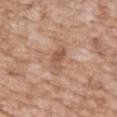Clinical impression: This lesion was catalogued during total-body skin photography and was not selected for biopsy. Acquisition and patient details: Located on the chest. A region of skin cropped from a whole-body photographic capture, roughly 15 mm wide. Automated image analysis of the tile measured a border-irregularity index near 3/10, a within-lesion color-variation index near 2.5/10, and radial color variation of about 1. A male patient about 60 years old. About 3 mm across.